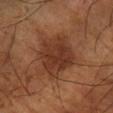Imaged during a routine full-body skin examination; the lesion was not biopsied and no histopathology is available. This is a cross-polarized tile. Cropped from a total-body skin-imaging series; the visible field is about 15 mm. An algorithmic analysis of the crop reported a lesion-to-skin contrast of about 8 (normalized; higher = more distinct). It also reported a border-irregularity rating of about 2.5/10 and radial color variation of about 1.5. A male patient, aged approximately 60. Located on the right forearm.